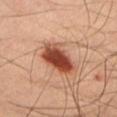follow-up: total-body-photography surveillance lesion; no biopsy | patient: male, roughly 45 years of age | diameter: ~5 mm (longest diameter) | anatomic site: the chest | acquisition: total-body-photography crop, ~15 mm field of view.